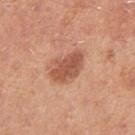Findings:
• follow-up: no biopsy performed (imaged during a skin exam)
• image: total-body-photography crop, ~15 mm field of view
• subject: male, about 30 years old
• site: the right upper arm